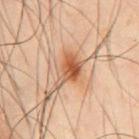Captured during whole-body skin photography for melanoma surveillance; the lesion was not biopsied.